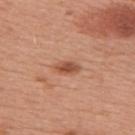Impression:
No biopsy was performed on this lesion — it was imaged during a full skin examination and was not determined to be concerning.
Acquisition and patient details:
The lesion is located on the right upper arm. A female patient aged 48 to 52. Measured at roughly 3 mm in maximum diameter. Imaged with white-light lighting. A roughly 15 mm field-of-view crop from a total-body skin photograph. Automated image analysis of the tile measured a lesion color around L≈52 a*≈25 b*≈33 in CIELAB and a lesion-to-skin contrast of about 8 (normalized; higher = more distinct). And it measured a border-irregularity rating of about 2/10, internal color variation of about 3 on a 0–10 scale, and a peripheral color-asymmetry measure near 1.5. It also reported an automated nevus-likeness rating near 85 out of 100 and lesion-presence confidence of about 100/100.